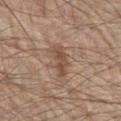Captured during whole-body skin photography for melanoma surveillance; the lesion was not biopsied.
From the chest.
A 15 mm close-up tile from a total-body photography series done for melanoma screening.
A male patient roughly 80 years of age.